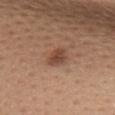{"biopsy_status": "not biopsied; imaged during a skin examination", "patient": {"sex": "female", "age_approx": 30}, "site": "upper back", "image": {"source": "total-body photography crop", "field_of_view_mm": 15}, "lesion_size": {"long_diameter_mm_approx": 2.5}}